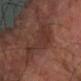Assessment:
No biopsy was performed on this lesion — it was imaged during a full skin examination and was not determined to be concerning.
Acquisition and patient details:
The lesion-visualizer software estimated an average lesion color of about L≈32 a*≈19 b*≈22 (CIELAB), about 5 CIELAB-L* units darker than the surrounding skin, and a normalized lesion–skin contrast near 5.5. The software also gave a within-lesion color-variation index near 3/10 and a peripheral color-asymmetry measure near 1. The software also gave an automated nevus-likeness rating near 5 out of 100 and lesion-presence confidence of about 100/100. Measured at roughly 6 mm in maximum diameter. The lesion is on the right forearm. Captured under cross-polarized illumination. A roughly 15 mm field-of-view crop from a total-body skin photograph. A male subject, about 70 years old.